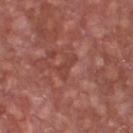| field | value |
|---|---|
| patient | male, in their mid- to late 60s |
| tile lighting | white-light illumination |
| TBP lesion metrics | a classifier nevus-likeness of about 0/100 |
| diameter | ~3 mm (longest diameter) |
| anatomic site | the front of the torso |
| imaging modality | ~15 mm tile from a whole-body skin photo |